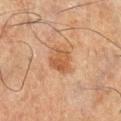biopsy_status: not biopsied; imaged during a skin examination
image:
  source: total-body photography crop
  field_of_view_mm: 15
lesion_size:
  long_diameter_mm_approx: 3.0
patient:
  sex: male
  age_approx: 65
lighting: cross-polarized
site: right lower leg
automated_metrics:
  eccentricity: 0.35
  shape_asymmetry: 0.2
  color_variation_0_10: 3.0
  peripheral_color_asymmetry: 1.0